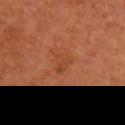No biopsy was performed on this lesion — it was imaged during a full skin examination and was not determined to be concerning. The lesion's longest dimension is about 2.5 mm. On the right upper arm. A female subject aged 53–57. A close-up tile cropped from a whole-body skin photograph, about 15 mm across.